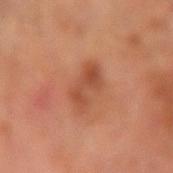Q: Is there a histopathology result?
A: total-body-photography surveillance lesion; no biopsy
Q: What did automated image analysis measure?
A: an outline eccentricity of about 0.8 (0 = round, 1 = elongated) and two-axis asymmetry of about 0.3; an average lesion color of about L≈49 a*≈27 b*≈34 (CIELAB), a lesion–skin lightness drop of about 9, and a lesion-to-skin contrast of about 6.5 (normalized; higher = more distinct); border irregularity of about 4 on a 0–10 scale, internal color variation of about 4 on a 0–10 scale, and a peripheral color-asymmetry measure near 1; a classifier nevus-likeness of about 5/100 and lesion-presence confidence of about 100/100
Q: Patient demographics?
A: male, aged 68 to 72
Q: What kind of image is this?
A: total-body-photography crop, ~15 mm field of view
Q: What is the anatomic site?
A: the arm
Q: Lesion size?
A: ≈4 mm
Q: How was the tile lit?
A: cross-polarized